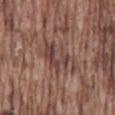No biopsy was performed on this lesion — it was imaged during a full skin examination and was not determined to be concerning.
Located on the mid back.
Captured under white-light illumination.
Cropped from a whole-body photographic skin survey; the tile spans about 15 mm.
Approximately 5.5 mm at its widest.
The subject is a male aged around 75.